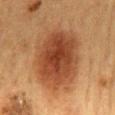Q: Was a biopsy performed?
A: catalogued during a skin exam; not biopsied
Q: How was this image acquired?
A: 15 mm crop, total-body photography
Q: Lesion location?
A: the mid back
Q: What are the patient's age and sex?
A: male, approximately 60 years of age
Q: How was the tile lit?
A: cross-polarized illumination